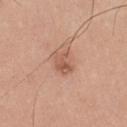Clinical summary: A male subject aged approximately 30. On the chest. A 15 mm close-up tile from a total-body photography series done for melanoma screening. The lesion's longest dimension is about 3 mm. Automated tile analysis of the lesion measured a within-lesion color-variation index near 4.5/10 and peripheral color asymmetry of about 2. The analysis additionally found an automated nevus-likeness rating near 45 out of 100.